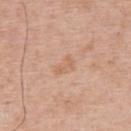{"biopsy_status": "not biopsied; imaged during a skin examination", "patient": {"sex": "male", "age_approx": 65}, "image": {"source": "total-body photography crop", "field_of_view_mm": 15}, "lesion_size": {"long_diameter_mm_approx": 3.0}, "site": "upper back"}